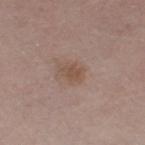notes: total-body-photography surveillance lesion; no biopsy | image-analysis metrics: a lesion area of about 4 mm² and an eccentricity of roughly 0.75; a lesion color around L≈50 a*≈17 b*≈26 in CIELAB and roughly 7 lightness units darker than nearby skin; a border-irregularity rating of about 3/10 and a peripheral color-asymmetry measure near 1; a nevus-likeness score of about 10/100 | patient: male, about 65 years old | site: the left thigh | imaging modality: total-body-photography crop, ~15 mm field of view | lighting: white-light.